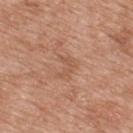follow-up: total-body-photography surveillance lesion; no biopsy | acquisition: total-body-photography crop, ~15 mm field of view | subject: male, in their 50s | location: the upper back.